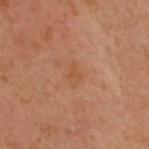<case>
<biopsy_status>not biopsied; imaged during a skin examination</biopsy_status>
<lesion_size>
  <long_diameter_mm_approx>2.5</long_diameter_mm_approx>
</lesion_size>
<image>
  <source>total-body photography crop</source>
  <field_of_view_mm>15</field_of_view_mm>
</image>
<site>head or neck</site>
<patient>
  <sex>male</sex>
  <age_approx>50</age_approx>
</patient>
<lighting>cross-polarized</lighting>
<automated_metrics>
  <area_mm2_approx>3.5</area_mm2_approx>
  <eccentricity>0.75</eccentricity>
  <lesion_detection_confidence_0_100>100</lesion_detection_confidence_0_100>
</automated_metrics>
</case>